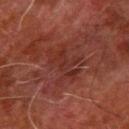A male subject, roughly 80 years of age.
Located on the arm.
The total-body-photography lesion software estimated an eccentricity of roughly 0.8 and two-axis asymmetry of about 0.4. The analysis additionally found a border-irregularity index near 8/10, a within-lesion color-variation index near 2.5/10, and radial color variation of about 1.
A region of skin cropped from a whole-body photographic capture, roughly 15 mm wide.
The tile uses cross-polarized illumination.
Approximately 5 mm at its widest.